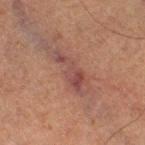workup: total-body-photography surveillance lesion; no biopsy
patient: female, aged approximately 60
anatomic site: the right thigh
acquisition: total-body-photography crop, ~15 mm field of view